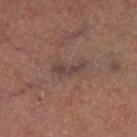  biopsy_status: not biopsied; imaged during a skin examination
  patient:
    sex: female
  site: left thigh
  lighting: cross-polarized
  lesion_size:
    long_diameter_mm_approx: 4.0
  image:
    source: total-body photography crop
    field_of_view_mm: 15